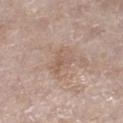The lesion was photographed on a routine skin check and not biopsied; there is no pathology result.
Longest diameter approximately 3 mm.
The tile uses white-light illumination.
The patient is a female in their mid- to late 70s.
The lesion is located on the right lower leg.
A lesion tile, about 15 mm wide, cut from a 3D total-body photograph.